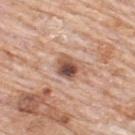Imaged during a routine full-body skin examination; the lesion was not biopsied and no histopathology is available.
On the upper back.
A region of skin cropped from a whole-body photographic capture, roughly 15 mm wide.
A male patient roughly 80 years of age.